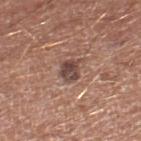biopsy status: imaged on a skin check; not biopsied
size: about 3 mm
patient: male, aged approximately 80
tile lighting: white-light illumination
image: total-body-photography crop, ~15 mm field of view
location: the right lower leg